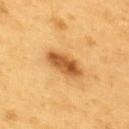Acquisition and patient details:
The total-body-photography lesion software estimated a border-irregularity rating of about 2.5/10, internal color variation of about 5.5 on a 0–10 scale, and a peripheral color-asymmetry measure near 2. The analysis additionally found a nevus-likeness score of about 90/100 and a detector confidence of about 100 out of 100 that the crop contains a lesion. This is a cross-polarized tile. The lesion's longest dimension is about 5 mm. A male subject, approximately 60 years of age. The lesion is located on the back. A close-up tile cropped from a whole-body skin photograph, about 15 mm across.